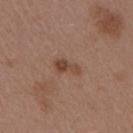Assessment:
The lesion was tiled from a total-body skin photograph and was not biopsied.
Context:
Located on the arm. A 15 mm crop from a total-body photograph taken for skin-cancer surveillance. The tile uses white-light illumination. The patient is a female aged 43–47.